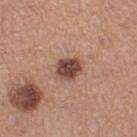{"lighting": "white-light", "patient": {"sex": "female", "age_approx": 35}, "image": {"source": "total-body photography crop", "field_of_view_mm": 15}, "site": "leg", "lesion_size": {"long_diameter_mm_approx": 3.0}, "automated_metrics": {"eccentricity": 0.4, "shape_asymmetry": 0.2, "nevus_likeness_0_100": 60, "lesion_detection_confidence_0_100": 100}}